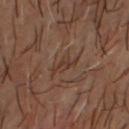patient:
  sex: male
  age_approx: 50
image:
  source: total-body photography crop
  field_of_view_mm: 15
lighting: cross-polarized
site: head or neck